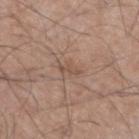follow-up: total-body-photography surveillance lesion; no biopsy | image: 15 mm crop, total-body photography | body site: the leg | lighting: white-light illumination | lesion diameter: about 2.5 mm | subject: male, aged 43–47 | automated metrics: a footprint of about 1.5 mm², an eccentricity of roughly 0.95, and a shape-asymmetry score of about 0.55 (0 = symmetric); a border-irregularity index near 6.5/10 and a within-lesion color-variation index near 0/10.